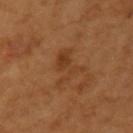The lesion was photographed on a routine skin check and not biopsied; there is no pathology result. This image is a 15 mm lesion crop taken from a total-body photograph. The total-body-photography lesion software estimated about 7 CIELAB-L* units darker than the surrounding skin. And it measured a border-irregularity index near 8.5/10 and a color-variation rating of about 2.5/10. On the left upper arm. The subject is a female aged 53–57. Captured under cross-polarized illumination. Approximately 4.5 mm at its widest.